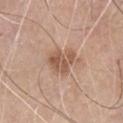Imaged during a routine full-body skin examination; the lesion was not biopsied and no histopathology is available.
The lesion-visualizer software estimated a normalized lesion–skin contrast near 7.
On the chest.
The patient is a male in their mid-70s.
Captured under white-light illumination.
This image is a 15 mm lesion crop taken from a total-body photograph.